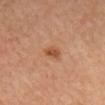biopsy_status: not biopsied; imaged during a skin examination
lesion_size:
  long_diameter_mm_approx: 2.5
image:
  source: total-body photography crop
  field_of_view_mm: 15
automated_metrics:
  border_irregularity_0_10: 2.5
  color_variation_0_10: 2.0
  peripheral_color_asymmetry: 0.5
  nevus_likeness_0_100: 85
site: head or neck
patient:
  sex: female
  age_approx: 40